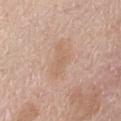The lesion was photographed on a routine skin check and not biopsied; there is no pathology result.
A close-up tile cropped from a whole-body skin photograph, about 15 mm across.
From the abdomen.
A female subject, about 75 years old.